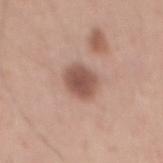Impression: This lesion was catalogued during total-body skin photography and was not selected for biopsy. Acquisition and patient details: An algorithmic analysis of the crop reported a lesion area of about 8 mm², an eccentricity of roughly 0.65, and a symmetry-axis asymmetry near 0.15. The analysis additionally found a border-irregularity index near 1.5/10, internal color variation of about 2.5 on a 0–10 scale, and peripheral color asymmetry of about 0.5. The recorded lesion diameter is about 3.5 mm. The lesion is on the mid back. A male subject, aged around 30. A close-up tile cropped from a whole-body skin photograph, about 15 mm across.